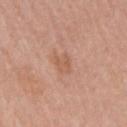Impression:
Recorded during total-body skin imaging; not selected for excision or biopsy.
Clinical summary:
The recorded lesion diameter is about 2.5 mm. A 15 mm close-up tile from a total-body photography series done for melanoma screening. From the right upper arm. Automated tile analysis of the lesion measured a nevus-likeness score of about 0/100. A female subject, in their mid- to late 50s.